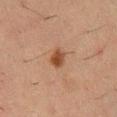Findings:
* biopsy status — total-body-photography surveillance lesion; no biopsy
* lighting — cross-polarized
* lesion size — ≈2.5 mm
* automated lesion analysis — a classifier nevus-likeness of about 95/100 and a lesion-detection confidence of about 100/100
* patient — male, about 50 years old
* site — the chest
* image source — ~15 mm crop, total-body skin-cancer survey